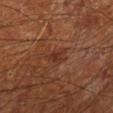– biopsy status · imaged on a skin check; not biopsied
– automated metrics · an average lesion color of about L≈31 a*≈22 b*≈28 (CIELAB), a lesion–skin lightness drop of about 6, and a normalized lesion–skin contrast near 5.5; a nevus-likeness score of about 0/100 and a detector confidence of about 100 out of 100 that the crop contains a lesion
– site · the left lower leg
– subject · male, in their mid-60s
– imaging modality · ~15 mm tile from a whole-body skin photo
– lesion size · about 3 mm